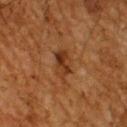Clinical impression:
Imaged during a routine full-body skin examination; the lesion was not biopsied and no histopathology is available.
Image and clinical context:
A male patient, aged 58–62. Located on the upper back. A 15 mm close-up tile from a total-body photography series done for melanoma screening. The lesion's longest dimension is about 3.5 mm. Automated tile analysis of the lesion measured a within-lesion color-variation index near 5.5/10 and radial color variation of about 2.5.